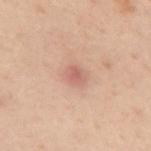site: the mid back
subject: male, aged approximately 50
automated metrics: a footprint of about 4 mm², an eccentricity of roughly 0.65, and two-axis asymmetry of about 0.25; a mean CIELAB color near L≈49 a*≈19 b*≈22 and roughly 7 lightness units darker than nearby skin; border irregularity of about 2 on a 0–10 scale, internal color variation of about 2.5 on a 0–10 scale, and peripheral color asymmetry of about 1
tile lighting: cross-polarized illumination
diameter: about 2.5 mm
image source: total-body-photography crop, ~15 mm field of view